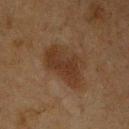follow-up = total-body-photography surveillance lesion; no biopsy
site = the front of the torso
image source = ~15 mm tile from a whole-body skin photo
patient = male, aged 73–77
lighting = cross-polarized illumination
lesion diameter = about 6 mm